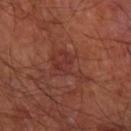Imaged during a routine full-body skin examination; the lesion was not biopsied and no histopathology is available. Cropped from a total-body skin-imaging series; the visible field is about 15 mm. The lesion is on the arm. The patient is a male approximately 65 years of age. About 5 mm across.